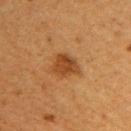{"image": {"source": "total-body photography crop", "field_of_view_mm": 15}, "site": "right upper arm", "patient": {"sex": "female", "age_approx": 40}, "lighting": "cross-polarized"}